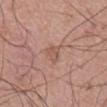follow-up: catalogued during a skin exam; not biopsied
body site: the mid back
lesion size: ≈2.5 mm
imaging modality: 15 mm crop, total-body photography
patient: male, about 55 years old
automated lesion analysis: a footprint of about 3 mm², an eccentricity of roughly 0.85, and a shape-asymmetry score of about 0.3 (0 = symmetric); border irregularity of about 3 on a 0–10 scale, a color-variation rating of about 1/10, and radial color variation of about 0; a nevus-likeness score of about 0/100